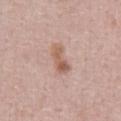This lesion was catalogued during total-body skin photography and was not selected for biopsy. A male patient aged 58 to 62. From the chest. Captured under white-light illumination. A roughly 15 mm field-of-view crop from a total-body skin photograph. The recorded lesion diameter is about 4 mm.